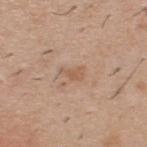workup: no biopsy performed (imaged during a skin exam) | subject: male, roughly 40 years of age | imaging modality: ~15 mm tile from a whole-body skin photo | site: the upper back | tile lighting: white-light illumination | size: ≈2.5 mm.